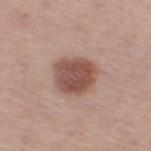notes: total-body-photography surveillance lesion; no biopsy
body site: the right thigh
subject: female, aged approximately 65
lesion size: ~4.5 mm (longest diameter)
automated lesion analysis: a shape eccentricity near 0.45 and a symmetry-axis asymmetry near 0.15; an average lesion color of about L≈50 a*≈21 b*≈24 (CIELAB) and a normalized border contrast of about 9.5; peripheral color asymmetry of about 1
imaging modality: 15 mm crop, total-body photography
illumination: white-light